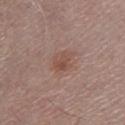biopsy status: total-body-photography surveillance lesion; no biopsy
location: the left lower leg
image source: 15 mm crop, total-body photography
patient: male, about 65 years old